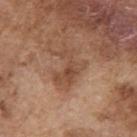This lesion was catalogued during total-body skin photography and was not selected for biopsy. From the right upper arm. A male patient, aged 73 to 77. The total-body-photography lesion software estimated an area of roughly 8 mm² and an eccentricity of roughly 0.8. The software also gave a lesion color around L≈48 a*≈20 b*≈31 in CIELAB and a normalized lesion–skin contrast near 6. It also reported a color-variation rating of about 3.5/10 and a peripheral color-asymmetry measure near 1. The software also gave lesion-presence confidence of about 100/100. A roughly 15 mm field-of-view crop from a total-body skin photograph. Imaged with white-light lighting. The recorded lesion diameter is about 4.5 mm.